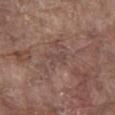The lesion was tiled from a total-body skin photograph and was not biopsied.
The subject is a male aged 78–82.
A 15 mm close-up tile from a total-body photography series done for melanoma screening.
The lesion is on the front of the torso.